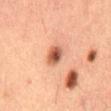follow-up — no biopsy performed (imaged during a skin exam)
image source — ~15 mm crop, total-body skin-cancer survey
automated metrics — an area of roughly 5.5 mm², an eccentricity of roughly 0.65, and two-axis asymmetry of about 0.25; an average lesion color of about L≈44 a*≈21 b*≈27 (CIELAB), roughly 13 lightness units darker than nearby skin, and a normalized lesion–skin contrast near 9.5
patient — male, aged 48–52
site — the lower back